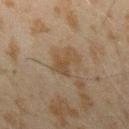notes: imaged on a skin check; not biopsied | location: the right forearm | patient: male, roughly 45 years of age | tile lighting: cross-polarized illumination | imaging modality: ~15 mm tile from a whole-body skin photo.